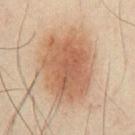workup: imaged on a skin check; not biopsied | body site: the chest | illumination: cross-polarized | image: total-body-photography crop, ~15 mm field of view | automated lesion analysis: an outline eccentricity of about 0.65 (0 = round, 1 = elongated) and a symmetry-axis asymmetry near 0.2; a border-irregularity index near 2.5/10, internal color variation of about 4.5 on a 0–10 scale, and a peripheral color-asymmetry measure near 1.5 | subject: male, about 50 years old.